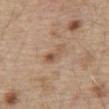Q: Was a biopsy performed?
A: total-body-photography surveillance lesion; no biopsy
Q: Lesion location?
A: the abdomen
Q: What is the lesion's diameter?
A: ~3 mm (longest diameter)
Q: What lighting was used for the tile?
A: white-light
Q: How was this image acquired?
A: 15 mm crop, total-body photography
Q: Patient demographics?
A: male, in their 70s